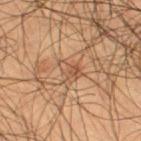Recorded during total-body skin imaging; not selected for excision or biopsy. The patient is a male aged 53–57. From the leg. Longest diameter approximately 3 mm. A 15 mm close-up extracted from a 3D total-body photography capture. Captured under cross-polarized illumination.